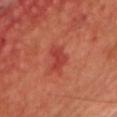notes: catalogued during a skin exam; not biopsied | subject: male, aged 48 to 52 | image: ~15 mm crop, total-body skin-cancer survey | site: the chest | automated lesion analysis: a shape eccentricity near 0.75 and a shape-asymmetry score of about 0.4 (0 = symmetric); an automated nevus-likeness rating near 15 out of 100 and a detector confidence of about 100 out of 100 that the crop contains a lesion | size: ≈3 mm.